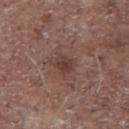Acquisition and patient details:
Measured at roughly 2.5 mm in maximum diameter. A close-up tile cropped from a whole-body skin photograph, about 15 mm across. Captured under white-light illumination. A male subject, roughly 70 years of age. The lesion is on the chest.